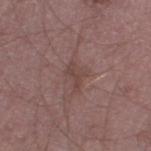Impression: Captured during whole-body skin photography for melanoma surveillance; the lesion was not biopsied.